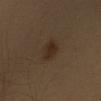The lesion was tiled from a total-body skin photograph and was not biopsied. Cropped from a whole-body photographic skin survey; the tile spans about 15 mm. The subject is a male in their 40s. This is a cross-polarized tile. The total-body-photography lesion software estimated an automated nevus-likeness rating near 90 out of 100 and a detector confidence of about 100 out of 100 that the crop contains a lesion. On the left upper arm.